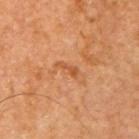Part of a total-body skin-imaging series; this lesion was reviewed on a skin check and was not flagged for biopsy. A region of skin cropped from a whole-body photographic capture, roughly 15 mm wide. Imaged with cross-polarized lighting. A patient approximately 65 years of age. The total-body-photography lesion software estimated a lesion area of about 2.5 mm², a shape eccentricity near 0.9, and a shape-asymmetry score of about 0.5 (0 = symmetric). And it measured a lesion color around L≈54 a*≈27 b*≈41 in CIELAB and a lesion–skin lightness drop of about 8. It also reported a nevus-likeness score of about 0/100 and lesion-presence confidence of about 100/100. Located on the right upper arm.